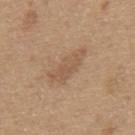Captured during whole-body skin photography for melanoma surveillance; the lesion was not biopsied. A male subject aged approximately 65. Longest diameter approximately 6 mm. The total-body-photography lesion software estimated a lesion color around L≈55 a*≈17 b*≈32 in CIELAB, about 7 CIELAB-L* units darker than the surrounding skin, and a normalized lesion–skin contrast near 5.5. And it measured a border-irregularity index near 4/10, internal color variation of about 2 on a 0–10 scale, and radial color variation of about 0.5. The analysis additionally found a nevus-likeness score of about 5/100 and a detector confidence of about 95 out of 100 that the crop contains a lesion. Imaged with white-light lighting. A close-up tile cropped from a whole-body skin photograph, about 15 mm across. The lesion is on the upper back.